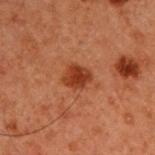Recorded during total-body skin imaging; not selected for excision or biopsy.
From the upper back.
A male subject, aged approximately 60.
An algorithmic analysis of the crop reported a lesion area of about 6 mm², an eccentricity of roughly 0.55, and two-axis asymmetry of about 0.25. It also reported an average lesion color of about L≈30 a*≈24 b*≈29 (CIELAB), roughly 9 lightness units darker than nearby skin, and a lesion-to-skin contrast of about 9 (normalized; higher = more distinct).
A 15 mm crop from a total-body photograph taken for skin-cancer surveillance.